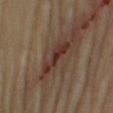Assessment:
Imaged during a routine full-body skin examination; the lesion was not biopsied and no histopathology is available.
Background:
Imaged with cross-polarized lighting. The recorded lesion diameter is about 5 mm. A 15 mm close-up extracted from a 3D total-body photography capture. A male subject, approximately 60 years of age. On the right upper arm.